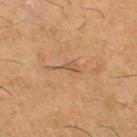Clinical impression:
This lesion was catalogued during total-body skin photography and was not selected for biopsy.
Acquisition and patient details:
The tile uses cross-polarized illumination. The subject is a male in their 60s. A roughly 15 mm field-of-view crop from a total-body skin photograph. From the right thigh. Longest diameter approximately 4 mm.